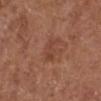Notes:
• workup · imaged on a skin check; not biopsied
• image · 15 mm crop, total-body photography
• diameter · ~2.5 mm (longest diameter)
• patient · male, aged around 75
• site · the right lower leg